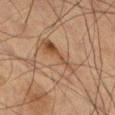Assessment: Part of a total-body skin-imaging series; this lesion was reviewed on a skin check and was not flagged for biopsy. Clinical summary: Located on the left thigh. The tile uses cross-polarized illumination. A male subject, about 65 years old. Longest diameter approximately 6 mm. A close-up tile cropped from a whole-body skin photograph, about 15 mm across. An algorithmic analysis of the crop reported an area of roughly 12 mm², a shape eccentricity near 0.9, and two-axis asymmetry of about 0.4. It also reported an average lesion color of about L≈41 a*≈15 b*≈27 (CIELAB) and about 6 CIELAB-L* units darker than the surrounding skin. The analysis additionally found a border-irregularity rating of about 6.5/10, a color-variation rating of about 6/10, and radial color variation of about 2. The analysis additionally found a detector confidence of about 95 out of 100 that the crop contains a lesion.